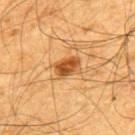follow-up = catalogued during a skin exam; not biopsied | image = ~15 mm tile from a whole-body skin photo | subject = male, about 65 years old | site = the upper back.